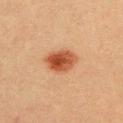<record>
  <biopsy_status>not biopsied; imaged during a skin examination</biopsy_status>
  <site>chest</site>
  <lesion_size>
    <long_diameter_mm_approx>4.0</long_diameter_mm_approx>
  </lesion_size>
  <patient>
    <sex>male</sex>
    <age_approx>40</age_approx>
  </patient>
  <automated_metrics>
    <cielab_L>43</cielab_L>
    <cielab_a>24</cielab_a>
    <cielab_b>32</cielab_b>
    <vs_skin_darker_L>13.0</vs_skin_darker_L>
    <border_irregularity_0_10>1.5</border_irregularity_0_10>
    <peripheral_color_asymmetry>1.5</peripheral_color_asymmetry>
    <lesion_detection_confidence_0_100>100</lesion_detection_confidence_0_100>
  </automated_metrics>
  <image>
    <source>total-body photography crop</source>
    <field_of_view_mm>15</field_of_view_mm>
  </image>
  <lighting>cross-polarized</lighting>
</record>